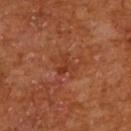{"patient": {"sex": "male", "age_approx": 65}, "image": {"source": "total-body photography crop", "field_of_view_mm": 15}, "site": "back"}